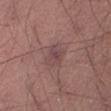Findings:
– follow-up — catalogued during a skin exam; not biopsied
– anatomic site — the right thigh
– lighting — white-light illumination
– image — ~15 mm tile from a whole-body skin photo
– patient — male, roughly 55 years of age